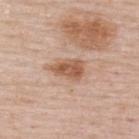biopsy_status: not biopsied; imaged during a skin examination
lesion_size:
  long_diameter_mm_approx: 4.0
lighting: white-light
image:
  source: total-body photography crop
  field_of_view_mm: 15
patient:
  sex: female
  age_approx: 50
site: back